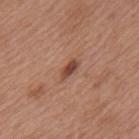The lesion was photographed on a routine skin check and not biopsied; there is no pathology result. Longest diameter approximately 2.5 mm. The patient is a female about 75 years old. Imaged with white-light lighting. The lesion is located on the upper back. A lesion tile, about 15 mm wide, cut from a 3D total-body photograph.